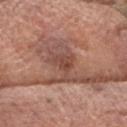The lesion was photographed on a routine skin check and not biopsied; there is no pathology result.
This is a white-light tile.
An algorithmic analysis of the crop reported a mean CIELAB color near L≈47 a*≈22 b*≈27, roughly 8 lightness units darker than nearby skin, and a normalized border contrast of about 6. The analysis additionally found a border-irregularity index near 6/10 and a peripheral color-asymmetry measure near 1.
Located on the head or neck.
Cropped from a whole-body photographic skin survey; the tile spans about 15 mm.
About 4 mm across.
A male patient, approximately 75 years of age.